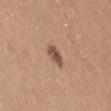Part of a total-body skin-imaging series; this lesion was reviewed on a skin check and was not flagged for biopsy. Located on the leg. The subject is a female roughly 40 years of age. Automated tile analysis of the lesion measured an average lesion color of about L≈51 a*≈20 b*≈27 (CIELAB), roughly 13 lightness units darker than nearby skin, and a normalized border contrast of about 9. The software also gave a nevus-likeness score of about 75/100 and a detector confidence of about 100 out of 100 that the crop contains a lesion. About 2.5 mm across. This image is a 15 mm lesion crop taken from a total-body photograph. This is a white-light tile.